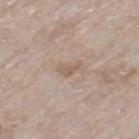Q: Was this lesion biopsied?
A: no biopsy performed (imaged during a skin exam)
Q: Where on the body is the lesion?
A: the left thigh
Q: Who is the patient?
A: female, aged around 75
Q: What is the imaging modality?
A: ~15 mm tile from a whole-body skin photo